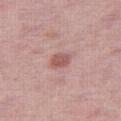follow-up: imaged on a skin check; not biopsied | illumination: white-light illumination | site: the right thigh | subject: female, about 60 years old | lesion size: ≈2.5 mm | imaging modality: total-body-photography crop, ~15 mm field of view | image-analysis metrics: an outline eccentricity of about 0.6 (0 = round, 1 = elongated); a classifier nevus-likeness of about 55/100 and lesion-presence confidence of about 100/100.